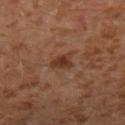biopsy status = no biopsy performed (imaged during a skin exam) | lighting = cross-polarized illumination | acquisition = total-body-photography crop, ~15 mm field of view | patient = male, in their 30s | lesion size = ≈3 mm | image-analysis metrics = a footprint of about 4.5 mm² and a symmetry-axis asymmetry near 0.45; border irregularity of about 4 on a 0–10 scale, a within-lesion color-variation index near 3/10, and radial color variation of about 1 | body site = the leg.